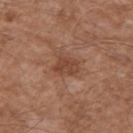{"biopsy_status": "not biopsied; imaged during a skin examination", "lesion_size": {"long_diameter_mm_approx": 3.0}, "image": {"source": "total-body photography crop", "field_of_view_mm": 15}, "automated_metrics": {"area_mm2_approx": 6.0, "eccentricity": 0.6, "shape_asymmetry": 0.25, "nevus_likeness_0_100": 0}, "site": "left upper arm", "lighting": "white-light", "patient": {"sex": "male", "age_approx": 60}}